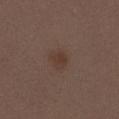Q: Is there a histopathology result?
A: imaged on a skin check; not biopsied
Q: Illumination type?
A: white-light
Q: How large is the lesion?
A: ~2.5 mm (longest diameter)
Q: What is the imaging modality?
A: ~15 mm crop, total-body skin-cancer survey
Q: Patient demographics?
A: female, aged 48–52
Q: What is the anatomic site?
A: the front of the torso